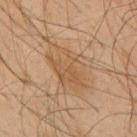Captured during whole-body skin photography for melanoma surveillance; the lesion was not biopsied. A 15 mm close-up extracted from a 3D total-body photography capture. The lesion's longest dimension is about 6.5 mm. A male patient, roughly 65 years of age. The lesion is on the upper back. Imaged with cross-polarized lighting.